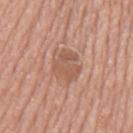Recorded during total-body skin imaging; not selected for excision or biopsy.
A male patient, aged approximately 75.
Automated tile analysis of the lesion measured an outline eccentricity of about 0.6 (0 = round, 1 = elongated) and a symmetry-axis asymmetry near 0.2.
The lesion is on the mid back.
Imaged with white-light lighting.
Cropped from a total-body skin-imaging series; the visible field is about 15 mm.
Longest diameter approximately 4 mm.